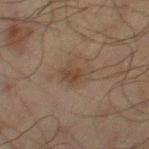Captured during whole-body skin photography for melanoma surveillance; the lesion was not biopsied. The tile uses cross-polarized illumination. A male patient, roughly 70 years of age. Approximately 3.5 mm at its widest. This image is a 15 mm lesion crop taken from a total-body photograph. The lesion is located on the right thigh.